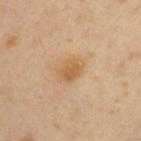Q: What is the anatomic site?
A: the left upper arm
Q: Who is the patient?
A: male, approximately 40 years of age
Q: Lesion size?
A: ~3.5 mm (longest diameter)
Q: What kind of image is this?
A: ~15 mm crop, total-body skin-cancer survey
Q: How was the tile lit?
A: cross-polarized illumination